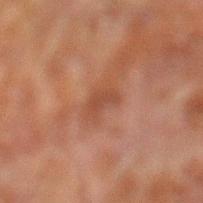This lesion was catalogued during total-body skin photography and was not selected for biopsy. A male patient, roughly 50 years of age. A 15 mm crop from a total-body photograph taken for skin-cancer surveillance. This is a cross-polarized tile. About 2.5 mm across. Automated image analysis of the tile measured an outline eccentricity of about 0.8 (0 = round, 1 = elongated) and a symmetry-axis asymmetry near 0.45. The lesion is on the left lower leg.